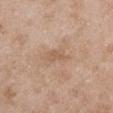Q: Was a biopsy performed?
A: imaged on a skin check; not biopsied
Q: What is the anatomic site?
A: the right upper arm
Q: Patient demographics?
A: male, in their 50s
Q: What kind of image is this?
A: total-body-photography crop, ~15 mm field of view
Q: What did automated image analysis measure?
A: a lesion–skin lightness drop of about 7; an automated nevus-likeness rating near 0 out of 100